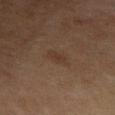Imaged during a routine full-body skin examination; the lesion was not biopsied and no histopathology is available. A female patient, in their 60s. Located on the left forearm. A close-up tile cropped from a whole-body skin photograph, about 15 mm across.